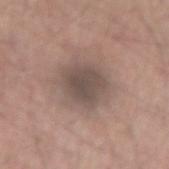follow-up: catalogued during a skin exam; not biopsied
image-analysis metrics: a footprint of about 13 mm², a shape eccentricity near 0.45, and a shape-asymmetry score of about 0.15 (0 = symmetric); a border-irregularity index near 1.5/10 and radial color variation of about 0.5; a classifier nevus-likeness of about 25/100 and lesion-presence confidence of about 100/100
subject: male, about 55 years old
body site: the right upper arm
diameter: ≈4.5 mm
image: ~15 mm crop, total-body skin-cancer survey